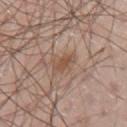Findings:
* notes — catalogued during a skin exam; not biopsied
* lighting — white-light illumination
* location — the left upper arm
* acquisition — total-body-photography crop, ~15 mm field of view
* patient — male, aged 63–67
* TBP lesion metrics — a footprint of about 3.5 mm², an outline eccentricity of about 0.9 (0 = round, 1 = elongated), and two-axis asymmetry of about 0.35; border irregularity of about 3.5 on a 0–10 scale, a color-variation rating of about 0.5/10, and radial color variation of about 0